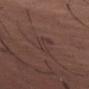Captured under white-light illumination. The lesion is on the abdomen. A region of skin cropped from a whole-body photographic capture, roughly 15 mm wide. A male patient approximately 45 years of age. The recorded lesion diameter is about 3 mm.